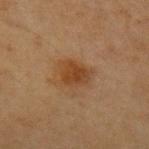biopsy_status: not biopsied; imaged during a skin examination
patient:
  sex: female
  age_approx: 40
site: left upper arm
image:
  source: total-body photography crop
  field_of_view_mm: 15
lighting: cross-polarized